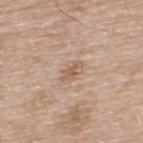Clinical impression: Recorded during total-body skin imaging; not selected for excision or biopsy.